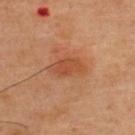Impression:
Part of a total-body skin-imaging series; this lesion was reviewed on a skin check and was not flagged for biopsy.
Image and clinical context:
Automated tile analysis of the lesion measured an average lesion color of about L≈47 a*≈26 b*≈34 (CIELAB), roughly 8 lightness units darker than nearby skin, and a lesion-to-skin contrast of about 6.5 (normalized; higher = more distinct). It also reported a classifier nevus-likeness of about 75/100 and lesion-presence confidence of about 100/100. The lesion is on the upper back. A male patient, aged 43 to 47. This image is a 15 mm lesion crop taken from a total-body photograph. Imaged with cross-polarized lighting.